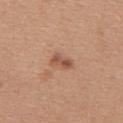Notes:
* notes — imaged on a skin check; not biopsied
* subject — female, approximately 45 years of age
* body site — the upper back
* image source — ~15 mm crop, total-body skin-cancer survey